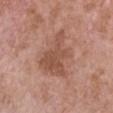Imaged during a routine full-body skin examination; the lesion was not biopsied and no histopathology is available. From the chest. This image is a 15 mm lesion crop taken from a total-body photograph. The tile uses white-light illumination. A female patient aged around 75. The total-body-photography lesion software estimated a nevus-likeness score of about 0/100 and a lesion-detection confidence of about 100/100. Approximately 6 mm at its widest.